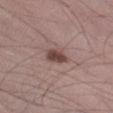Background: Located on the leg. The total-body-photography lesion software estimated an area of roughly 5 mm², an eccentricity of roughly 0.7, and two-axis asymmetry of about 0.25. It also reported a within-lesion color-variation index near 3/10 and a peripheral color-asymmetry measure near 1. This is a white-light tile. A region of skin cropped from a whole-body photographic capture, roughly 15 mm wide. A male subject aged around 35. Approximately 3 mm at its widest.